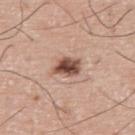Case summary:
• biopsy status — no biopsy performed (imaged during a skin exam)
• imaging modality — ~15 mm tile from a whole-body skin photo
• illumination — white-light illumination
• subject — male, aged 73–77
• lesion diameter — ≈3.5 mm
• TBP lesion metrics — a border-irregularity rating of about 2.5/10
• location — the upper back